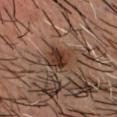Impression: Captured during whole-body skin photography for melanoma surveillance; the lesion was not biopsied. Clinical summary: A male subject, in their 50s. The lesion is located on the head or neck. Cropped from a total-body skin-imaging series; the visible field is about 15 mm. Imaged with cross-polarized lighting.